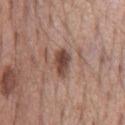image = ~15 mm tile from a whole-body skin photo
lesion size = ~3.5 mm (longest diameter)
tile lighting = white-light
location = the chest
subject = male, roughly 55 years of age